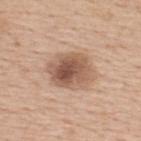Assessment:
The lesion was photographed on a routine skin check and not biopsied; there is no pathology result.
Context:
This is a white-light tile. Longest diameter approximately 5 mm. Automated tile analysis of the lesion measured an outline eccentricity of about 0.65 (0 = round, 1 = elongated). And it measured a lesion color around L≈55 a*≈19 b*≈29 in CIELAB and a lesion-to-skin contrast of about 9 (normalized; higher = more distinct). The analysis additionally found a classifier nevus-likeness of about 85/100 and a detector confidence of about 100 out of 100 that the crop contains a lesion. A region of skin cropped from a whole-body photographic capture, roughly 15 mm wide. A male patient roughly 60 years of age. Located on the upper back.